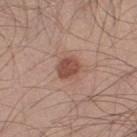The lesion was photographed on a routine skin check and not biopsied; there is no pathology result. A male subject in their 30s. Cropped from a total-body skin-imaging series; the visible field is about 15 mm. Located on the right thigh. The recorded lesion diameter is about 2.5 mm. Captured under white-light illumination.